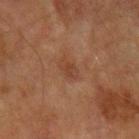- workup · imaged on a skin check; not biopsied